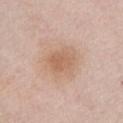| field | value |
|---|---|
| biopsy status | catalogued during a skin exam; not biopsied |
| body site | the chest |
| imaging modality | ~15 mm tile from a whole-body skin photo |
| lesion diameter | ~3.5 mm (longest diameter) |
| tile lighting | white-light illumination |
| subject | female, in their mid-60s |